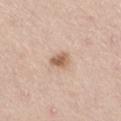| key | value |
|---|---|
| biopsy status | catalogued during a skin exam; not biopsied |
| body site | the leg |
| diameter | ≈2.5 mm |
| patient | male, aged 63 to 67 |
| acquisition | 15 mm crop, total-body photography |
| lighting | white-light illumination |
| TBP lesion metrics | a lesion color around L≈61 a*≈19 b*≈31 in CIELAB, about 13 CIELAB-L* units darker than the surrounding skin, and a normalized lesion–skin contrast near 8.5; a border-irregularity index near 2.5/10, a within-lesion color-variation index near 2.5/10, and peripheral color asymmetry of about 1 |